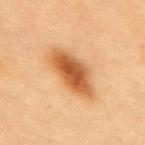Assessment: The lesion was tiled from a total-body skin photograph and was not biopsied. Image and clinical context: Located on the back. Cropped from a total-body skin-imaging series; the visible field is about 15 mm. A female patient, aged around 80.